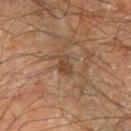Part of a total-body skin-imaging series; this lesion was reviewed on a skin check and was not flagged for biopsy.
A male patient, aged 68–72.
The total-body-photography lesion software estimated a border-irregularity rating of about 2.5/10, internal color variation of about 1.5 on a 0–10 scale, and a peripheral color-asymmetry measure near 0.5. The software also gave a classifier nevus-likeness of about 5/100 and lesion-presence confidence of about 100/100.
On the left forearm.
A close-up tile cropped from a whole-body skin photograph, about 15 mm across.
Longest diameter approximately 2.5 mm.